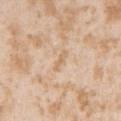Part of a total-body skin-imaging series; this lesion was reviewed on a skin check and was not flagged for biopsy. On the left upper arm. The patient is a female in their mid- to late 20s. Captured under white-light illumination. A roughly 15 mm field-of-view crop from a total-body skin photograph. Approximately 3 mm at its widest.